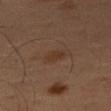Impression: The lesion was tiled from a total-body skin photograph and was not biopsied. Image and clinical context: A 15 mm close-up extracted from a 3D total-body photography capture. From the right thigh. The patient is a male aged 48–52. The lesion-visualizer software estimated a shape-asymmetry score of about 0.3 (0 = symmetric). The analysis additionally found a border-irregularity index near 2.5/10, internal color variation of about 1.5 on a 0–10 scale, and a peripheral color-asymmetry measure near 0.5. The analysis additionally found a lesion-detection confidence of about 100/100.